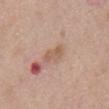From the chest.
A 15 mm close-up extracted from a 3D total-body photography capture.
A male subject, roughly 70 years of age.
Approximately 3 mm at its widest.
This is a white-light tile.